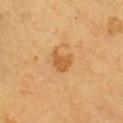{
  "biopsy_status": "not biopsied; imaged during a skin examination",
  "lighting": "cross-polarized",
  "image": {
    "source": "total-body photography crop",
    "field_of_view_mm": 15
  },
  "patient": {
    "sex": "male",
    "age_approx": 60
  },
  "site": "chest",
  "automated_metrics": {
    "area_mm2_approx": 4.0,
    "eccentricity": 0.7,
    "shape_asymmetry": 0.4,
    "nevus_likeness_0_100": 55,
    "lesion_detection_confidence_0_100": 100
  }
}